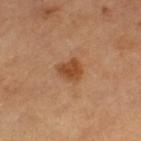Assessment: The lesion was tiled from a total-body skin photograph and was not biopsied. Context: About 3 mm across. An algorithmic analysis of the crop reported a lesion area of about 6 mm². And it measured an average lesion color of about L≈49 a*≈24 b*≈38 (CIELAB) and about 10 CIELAB-L* units darker than the surrounding skin. The analysis additionally found a color-variation rating of about 2.5/10 and peripheral color asymmetry of about 1. The software also gave a classifier nevus-likeness of about 90/100 and a lesion-detection confidence of about 100/100. The subject is a female aged approximately 60. A roughly 15 mm field-of-view crop from a total-body skin photograph. The lesion is on the left thigh. This is a cross-polarized tile.